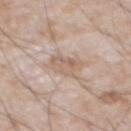<record>
<biopsy_status>not biopsied; imaged during a skin examination</biopsy_status>
<site>front of the torso</site>
<lighting>white-light</lighting>
<lesion_size>
  <long_diameter_mm_approx>3.0</long_diameter_mm_approx>
</lesion_size>
<automated_metrics>
  <area_mm2_approx>7.5</area_mm2_approx>
  <eccentricity>0.5</eccentricity>
  <nevus_likeness_0_100>0</nevus_likeness_0_100>
  <lesion_detection_confidence_0_100>100</lesion_detection_confidence_0_100>
</automated_metrics>
<patient>
  <sex>male</sex>
  <age_approx>65</age_approx>
</patient>
<image>
  <source>total-body photography crop</source>
  <field_of_view_mm>15</field_of_view_mm>
</image>
</record>